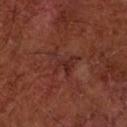<case>
  <biopsy_status>not biopsied; imaged during a skin examination</biopsy_status>
  <lesion_size>
    <long_diameter_mm_approx>4.5</long_diameter_mm_approx>
  </lesion_size>
  <site>arm</site>
  <image>
    <source>total-body photography crop</source>
    <field_of_view_mm>15</field_of_view_mm>
  </image>
  <patient>
    <sex>male</sex>
    <age_approx>65</age_approx>
  </patient>
</case>